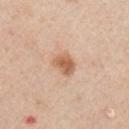Impression:
Imaged during a routine full-body skin examination; the lesion was not biopsied and no histopathology is available.
Clinical summary:
A 15 mm close-up extracted from a 3D total-body photography capture. Imaged with white-light lighting. A male patient, aged 43 to 47. The lesion-visualizer software estimated a color-variation rating of about 3.5/10 and peripheral color asymmetry of about 1. The lesion is on the chest. Longest diameter approximately 2.5 mm.